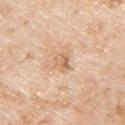notes = imaged on a skin check; not biopsied
location = the right upper arm
imaging modality = ~15 mm tile from a whole-body skin photo
subject = male, in their mid-70s
illumination = white-light illumination